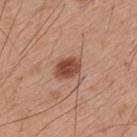notes: no biopsy performed (imaged during a skin exam); subject: male, aged 28–32; image source: ~15 mm crop, total-body skin-cancer survey; TBP lesion metrics: a border-irregularity index near 1.5/10, a color-variation rating of about 3.5/10, and peripheral color asymmetry of about 1; illumination: white-light; lesion diameter: ≈3 mm; anatomic site: the upper back.